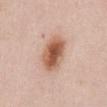{"biopsy_status": "not biopsied; imaged during a skin examination", "patient": {"sex": "female", "age_approx": 40}, "lesion_size": {"long_diameter_mm_approx": 5.0}, "site": "abdomen", "image": {"source": "total-body photography crop", "field_of_view_mm": 15}}